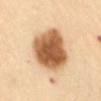Impression:
The lesion was tiled from a total-body skin photograph and was not biopsied.
Acquisition and patient details:
From the abdomen. Cropped from a total-body skin-imaging series; the visible field is about 15 mm. The subject is a female approximately 60 years of age. The lesion's longest dimension is about 6.5 mm. Imaged with cross-polarized lighting.